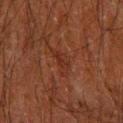workup: total-body-photography surveillance lesion; no biopsy
image-analysis metrics: a footprint of about 4 mm², a shape eccentricity near 0.9, and two-axis asymmetry of about 0.55; a mean CIELAB color near L≈23 a*≈20 b*≈24 and a normalized lesion–skin contrast near 5
imaging modality: total-body-photography crop, ~15 mm field of view
patient: male, approximately 60 years of age
lesion diameter: ~3.5 mm (longest diameter)
site: the right forearm
tile lighting: cross-polarized